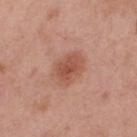<case>
<biopsy_status>not biopsied; imaged during a skin examination</biopsy_status>
<lesion_size>
  <long_diameter_mm_approx>4.5</long_diameter_mm_approx>
</lesion_size>
<patient>
  <sex>male</sex>
  <age_approx>55</age_approx>
</patient>
<image>
  <source>total-body photography crop</source>
  <field_of_view_mm>15</field_of_view_mm>
</image>
<site>back</site>
<automated_metrics>
  <area_mm2_approx>10.0</area_mm2_approx>
  <eccentricity>0.75</eccentricity>
  <shape_asymmetry>0.15</shape_asymmetry>
  <cielab_L>53</cielab_L>
  <cielab_a>24</cielab_a>
  <cielab_b>30</cielab_b>
  <vs_skin_darker_L>9.0</vs_skin_darker_L>
  <vs_skin_contrast_norm>7.0</vs_skin_contrast_norm>
  <border_irregularity_0_10>2.0</border_irregularity_0_10>
  <peripheral_color_asymmetry>1.0</peripheral_color_asymmetry>
</automated_metrics>
</case>